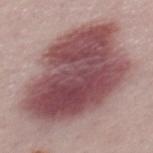{"biopsy_status": "not biopsied; imaged during a skin examination"}